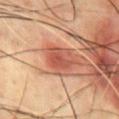Assessment: Imaged during a routine full-body skin examination; the lesion was not biopsied and no histopathology is available. Context: A region of skin cropped from a whole-body photographic capture, roughly 15 mm wide. Located on the chest. A male patient, about 70 years old. An algorithmic analysis of the crop reported a lesion color around L≈52 a*≈29 b*≈31 in CIELAB and a lesion–skin lightness drop of about 10. The tile uses cross-polarized illumination.